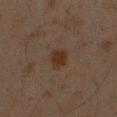Q: Was a biopsy performed?
A: imaged on a skin check; not biopsied
Q: Lesion location?
A: the arm
Q: Who is the patient?
A: male, aged 43–47
Q: Automated lesion metrics?
A: a lesion color around L≈24 a*≈13 b*≈22 in CIELAB and roughly 6 lightness units darker than nearby skin; a within-lesion color-variation index near 1.5/10 and radial color variation of about 0.5
Q: Lesion size?
A: ~2.5 mm (longest diameter)
Q: What is the imaging modality?
A: total-body-photography crop, ~15 mm field of view
Q: Illumination type?
A: cross-polarized